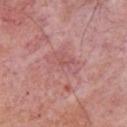Impression: Imaged during a routine full-body skin examination; the lesion was not biopsied and no histopathology is available. Context: Located on the front of the torso. Cropped from a whole-body photographic skin survey; the tile spans about 15 mm. The subject is a male in their mid-70s. Automated tile analysis of the lesion measured a border-irregularity index near 3/10, a within-lesion color-variation index near 0/10, and radial color variation of about 0. The analysis additionally found a detector confidence of about 85 out of 100 that the crop contains a lesion. The tile uses white-light illumination.